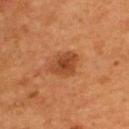Findings:
• biopsy status: imaged on a skin check; not biopsied
• patient: male, in their mid- to late 50s
• acquisition: ~15 mm crop, total-body skin-cancer survey
• lighting: cross-polarized illumination
• lesion size: ~4 mm (longest diameter)
• anatomic site: the upper back